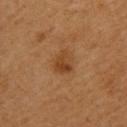biopsy status: no biopsy performed (imaged during a skin exam)
TBP lesion metrics: a lesion color around L≈37 a*≈20 b*≈33 in CIELAB, about 7 CIELAB-L* units darker than the surrounding skin, and a lesion-to-skin contrast of about 7 (normalized; higher = more distinct)
diameter: ≈3 mm
subject: male, roughly 55 years of age
image source: 15 mm crop, total-body photography
anatomic site: the upper back